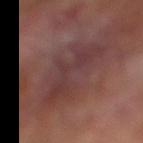Clinical impression:
This lesion was catalogued during total-body skin photography and was not selected for biopsy.
Context:
Cropped from a total-body skin-imaging series; the visible field is about 15 mm. An algorithmic analysis of the crop reported a border-irregularity index near 8.5/10 and peripheral color asymmetry of about 1.5. The software also gave an automated nevus-likeness rating near 0 out of 100 and lesion-presence confidence of about 85/100. The subject is a male about 70 years old. On the right lower leg.